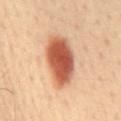<case>
<biopsy_status>not biopsied; imaged during a skin examination</biopsy_status>
<lighting>cross-polarized</lighting>
<image>
  <source>total-body photography crop</source>
  <field_of_view_mm>15</field_of_view_mm>
</image>
<lesion_size>
  <long_diameter_mm_approx>6.5</long_diameter_mm_approx>
</lesion_size>
<patient>
  <sex>male</sex>
  <age_approx>60</age_approx>
</patient>
<site>mid back</site>
<automated_metrics>
  <area_mm2_approx>19.0</area_mm2_approx>
  <eccentricity>0.85</eccentricity>
  <shape_asymmetry>0.15</shape_asymmetry>
  <cielab_L>57</cielab_L>
  <cielab_a>28</cielab_a>
  <cielab_b>34</cielab_b>
  <vs_skin_darker_L>19.0</vs_skin_darker_L>
  <vs_skin_contrast_norm>11.5</vs_skin_contrast_norm>
  <border_irregularity_0_10>2.0</border_irregularity_0_10>
  <color_variation_0_10>6.0</color_variation_0_10>
  <peripheral_color_asymmetry>1.5</peripheral_color_asymmetry>
  <nevus_likeness_0_100>100</nevus_likeness_0_100>
  <lesion_detection_confidence_0_100>100</lesion_detection_confidence_0_100>
</automated_metrics>
</case>